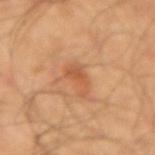follow-up = no biopsy performed (imaged during a skin exam); location = the left forearm; patient = male, approximately 55 years of age; acquisition = ~15 mm crop, total-body skin-cancer survey; lesion size = ~3 mm (longest diameter); tile lighting = cross-polarized.